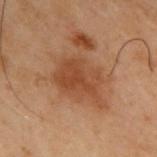workup: total-body-photography surveillance lesion; no biopsy
diameter: ~5.5 mm (longest diameter)
body site: the back
patient: male, aged 53 to 57
image source: 15 mm crop, total-body photography
TBP lesion metrics: a footprint of about 15 mm² and an outline eccentricity of about 0.7 (0 = round, 1 = elongated); a lesion color around L≈37 a*≈20 b*≈29 in CIELAB, roughly 7 lightness units darker than nearby skin, and a lesion-to-skin contrast of about 7 (normalized; higher = more distinct); a nevus-likeness score of about 5/100 and lesion-presence confidence of about 100/100
illumination: cross-polarized